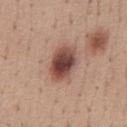notes: no biopsy performed (imaged during a skin exam)
subject: male, aged 38–42
acquisition: ~15 mm tile from a whole-body skin photo
size: about 5 mm
location: the abdomen